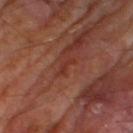<record>
  <biopsy_status>not biopsied; imaged during a skin examination</biopsy_status>
  <lesion_size>
    <long_diameter_mm_approx>2.5</long_diameter_mm_approx>
  </lesion_size>
  <image>
    <source>total-body photography crop</source>
    <field_of_view_mm>15</field_of_view_mm>
  </image>
  <site>right thigh</site>
  <patient>
    <sex>male</sex>
    <age_approx>70</age_approx>
  </patient>
</record>